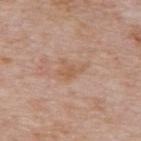Captured during whole-body skin photography for melanoma surveillance; the lesion was not biopsied.
The patient is a male in their mid-70s.
The tile uses white-light illumination.
On the back.
Measured at roughly 3 mm in maximum diameter.
A lesion tile, about 15 mm wide, cut from a 3D total-body photograph.
The total-body-photography lesion software estimated radial color variation of about 0.5.